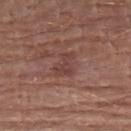Notes:
* follow-up · imaged on a skin check; not biopsied
* subject · male, aged around 85 or older
* illumination · white-light illumination
* anatomic site · the left upper arm
* TBP lesion metrics · a lesion area of about 6 mm², an eccentricity of roughly 0.75, and two-axis asymmetry of about 0.45; a mean CIELAB color near L≈42 a*≈21 b*≈23, a lesion–skin lightness drop of about 6, and a normalized lesion–skin contrast near 5; a classifier nevus-likeness of about 0/100 and a detector confidence of about 100 out of 100 that the crop contains a lesion
* image · ~15 mm tile from a whole-body skin photo